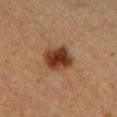Recorded during total-body skin imaging; not selected for excision or biopsy. Automated image analysis of the tile measured a lesion color around L≈31 a*≈19 b*≈27 in CIELAB. A 15 mm close-up tile from a total-body photography series done for melanoma screening. Imaged with cross-polarized lighting. A female subject aged around 40. The lesion's longest dimension is about 3.5 mm. On the front of the torso.